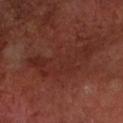The lesion was photographed on a routine skin check and not biopsied; there is no pathology result.
The total-body-photography lesion software estimated a border-irregularity rating of about 9.5/10, internal color variation of about 2.5 on a 0–10 scale, and peripheral color asymmetry of about 0.5.
Captured under cross-polarized illumination.
A close-up tile cropped from a whole-body skin photograph, about 15 mm across.
A male subject in their mid- to late 60s.
The lesion is located on the left forearm.
About 9 mm across.